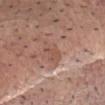Q: Was a biopsy performed?
A: catalogued during a skin exam; not biopsied
Q: Where on the body is the lesion?
A: the chest
Q: Who is the patient?
A: male, about 60 years old
Q: What is the imaging modality?
A: 15 mm crop, total-body photography